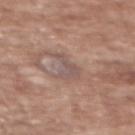The lesion was tiled from a total-body skin photograph and was not biopsied.
Measured at roughly 3.5 mm in maximum diameter.
The lesion is on the back.
Captured under white-light illumination.
A female patient approximately 75 years of age.
Automated image analysis of the tile measured an area of roughly 4 mm² and an eccentricity of roughly 0.85. It also reported a mean CIELAB color near L≈52 a*≈16 b*≈21, roughly 6 lightness units darker than nearby skin, and a normalized border contrast of about 5.5. And it measured a border-irregularity index near 8/10, a within-lesion color-variation index near 0/10, and a peripheral color-asymmetry measure near 0.
Cropped from a total-body skin-imaging series; the visible field is about 15 mm.